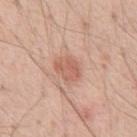The lesion was photographed on a routine skin check and not biopsied; there is no pathology result.
Automated image analysis of the tile measured roughly 9 lightness units darker than nearby skin and a lesion-to-skin contrast of about 6 (normalized; higher = more distinct).
Cropped from a total-body skin-imaging series; the visible field is about 15 mm.
A male patient aged 53 to 57.
About 3 mm across.
This is a white-light tile.
The lesion is located on the chest.